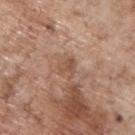Image and clinical context:
This is a white-light tile. The recorded lesion diameter is about 2.5 mm. From the back. The subject is a male aged around 70. The total-body-photography lesion software estimated an automated nevus-likeness rating near 0 out of 100. A roughly 15 mm field-of-view crop from a total-body skin photograph.